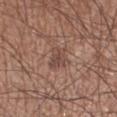| feature | finding |
|---|---|
| notes | total-body-photography surveillance lesion; no biopsy |
| diameter | ~3.5 mm (longest diameter) |
| image | 15 mm crop, total-body photography |
| illumination | white-light |
| automated lesion analysis | a lesion area of about 6 mm² and an outline eccentricity of about 0.7 (0 = round, 1 = elongated) |
| patient | male, aged approximately 55 |
| body site | the left lower leg |